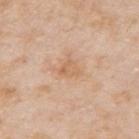Q: Was this lesion biopsied?
A: catalogued during a skin exam; not biopsied
Q: What is the imaging modality?
A: 15 mm crop, total-body photography
Q: What is the lesion's diameter?
A: ≈2.5 mm
Q: Where on the body is the lesion?
A: the arm
Q: Who is the patient?
A: male, in their mid-70s
Q: What did automated image analysis measure?
A: an area of roughly 4 mm² and an outline eccentricity of about 0.45 (0 = round, 1 = elongated); a lesion–skin lightness drop of about 7 and a normalized border contrast of about 5; a nevus-likeness score of about 0/100 and lesion-presence confidence of about 100/100
Q: What lighting was used for the tile?
A: white-light